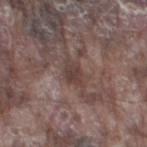Clinical impression:
The lesion was tiled from a total-body skin photograph and was not biopsied.
Clinical summary:
Automated image analysis of the tile measured a footprint of about 5 mm², a shape eccentricity near 0.7, and a shape-asymmetry score of about 0.3 (0 = symmetric). It also reported a lesion color around L≈40 a*≈16 b*≈19 in CIELAB, a lesion–skin lightness drop of about 8, and a normalized lesion–skin contrast near 7. The lesion's longest dimension is about 3 mm. From the left thigh. A male subject, approximately 75 years of age. A 15 mm close-up extracted from a 3D total-body photography capture. Imaged with white-light lighting.